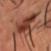Captured during whole-body skin photography for melanoma surveillance; the lesion was not biopsied. This is a cross-polarized tile. A region of skin cropped from a whole-body photographic capture, roughly 15 mm wide. A male patient aged 33 to 37. Measured at roughly 16 mm in maximum diameter. On the head or neck.